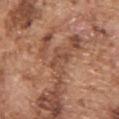follow-up: no biopsy performed (imaged during a skin exam); subject: male, aged around 75; lesion diameter: ~2.5 mm (longest diameter); tile lighting: white-light illumination; acquisition: total-body-photography crop, ~15 mm field of view; site: the upper back.